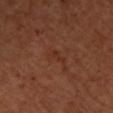<tbp_lesion>
  <biopsy_status>not biopsied; imaged during a skin examination</biopsy_status>
  <automated_metrics>
    <area_mm2_approx>3.0</area_mm2_approx>
    <eccentricity>0.9</eccentricity>
    <cielab_L>32</cielab_L>
    <cielab_a>23</cielab_a>
    <cielab_b>29</cielab_b>
    <vs_skin_darker_L>5.0</vs_skin_darker_L>
    <vs_skin_contrast_norm>5.0</vs_skin_contrast_norm>
  </automated_metrics>
  <patient>
    <sex>female</sex>
    <age_approx>30</age_approx>
  </patient>
  <lighting>cross-polarized</lighting>
  <site>upper back</site>
  <image>
    <source>total-body photography crop</source>
    <field_of_view_mm>15</field_of_view_mm>
  </image>
</tbp_lesion>